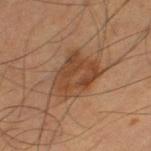| key | value |
|---|---|
| notes | no biopsy performed (imaged during a skin exam) |
| automated lesion analysis | a footprint of about 19 mm², a shape eccentricity near 0.75, and two-axis asymmetry of about 0.3; a classifier nevus-likeness of about 30/100 and a lesion-detection confidence of about 100/100 |
| acquisition | total-body-photography crop, ~15 mm field of view |
| body site | the right thigh |
| lighting | cross-polarized illumination |
| patient | male, roughly 60 years of age |